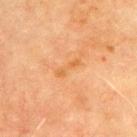Assessment:
This lesion was catalogued during total-body skin photography and was not selected for biopsy.
Image and clinical context:
On the chest. A male subject, aged around 70. This image is a 15 mm lesion crop taken from a total-body photograph.